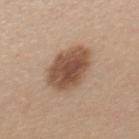Impression:
The lesion was photographed on a routine skin check and not biopsied; there is no pathology result.
Clinical summary:
The tile uses white-light illumination. Located on the upper back. The patient is a female aged 38 to 42. The lesion's longest dimension is about 5.5 mm. A lesion tile, about 15 mm wide, cut from a 3D total-body photograph.